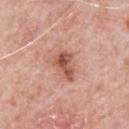No biopsy was performed on this lesion — it was imaged during a full skin examination and was not determined to be concerning.
The lesion is on the chest.
A male subject about 80 years old.
The total-body-photography lesion software estimated a color-variation rating of about 7/10 and radial color variation of about 2.5. The analysis additionally found an automated nevus-likeness rating near 55 out of 100 and lesion-presence confidence of about 100/100.
A region of skin cropped from a whole-body photographic capture, roughly 15 mm wide.
The lesion's longest dimension is about 3.5 mm.